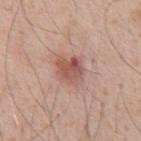Imaged during a routine full-body skin examination; the lesion was not biopsied and no histopathology is available. The lesion is located on the front of the torso. A close-up tile cropped from a whole-body skin photograph, about 15 mm across. A male patient in their mid- to late 50s. Approximately 3.5 mm at its widest. The total-body-photography lesion software estimated roughly 10 lightness units darker than nearby skin. The software also gave a nevus-likeness score of about 90/100.